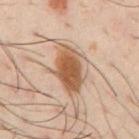{
  "biopsy_status": "not biopsied; imaged during a skin examination",
  "patient": {
    "sex": "male",
    "age_approx": 40
  },
  "image": {
    "source": "total-body photography crop",
    "field_of_view_mm": 15
  },
  "lighting": "cross-polarized",
  "lesion_size": {
    "long_diameter_mm_approx": 6.0
  },
  "site": "chest"
}